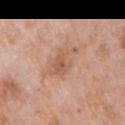Case summary:
– subject · female, roughly 70 years of age
– acquisition · ~15 mm tile from a whole-body skin photo
– location · the right upper arm